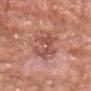workup=no biopsy performed (imaged during a skin exam)
patient=male, roughly 80 years of age
size=≈5.5 mm
imaging modality=~15 mm tile from a whole-body skin photo
image-analysis metrics=an eccentricity of roughly 0.8; a lesion–skin lightness drop of about 9 and a normalized border contrast of about 6; a nevus-likeness score of about 0/100 and lesion-presence confidence of about 100/100
tile lighting=white-light
site=the head or neck